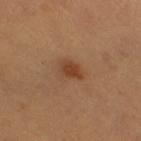Q: Was this lesion biopsied?
A: no biopsy performed (imaged during a skin exam)
Q: What did automated image analysis measure?
A: a lesion area of about 4.5 mm², an eccentricity of roughly 0.7, and a shape-asymmetry score of about 0.25 (0 = symmetric); internal color variation of about 2 on a 0–10 scale
Q: Who is the patient?
A: female, roughly 45 years of age
Q: How was this image acquired?
A: ~15 mm tile from a whole-body skin photo
Q: Where on the body is the lesion?
A: the left leg